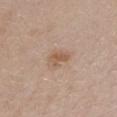Captured during whole-body skin photography for melanoma surveillance; the lesion was not biopsied.